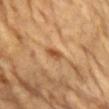Captured during whole-body skin photography for melanoma surveillance; the lesion was not biopsied. This is a cross-polarized tile. From the front of the torso. A 15 mm crop from a total-body photograph taken for skin-cancer surveillance. About 2.5 mm across. A female subject about 60 years old.